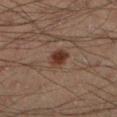notes=total-body-photography surveillance lesion; no biopsy.